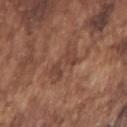Clinical impression:
No biopsy was performed on this lesion — it was imaged during a full skin examination and was not determined to be concerning.
Clinical summary:
Captured under white-light illumination. Cropped from a total-body skin-imaging series; the visible field is about 15 mm. Located on the right upper arm. A male patient, aged 73–77. Approximately 4 mm at its widest.